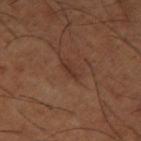Q: Is there a histopathology result?
A: catalogued during a skin exam; not biopsied
Q: What is the lesion's diameter?
A: ≈2.5 mm
Q: How was this image acquired?
A: ~15 mm crop, total-body skin-cancer survey
Q: What lighting was used for the tile?
A: cross-polarized
Q: Patient demographics?
A: male, about 65 years old
Q: Lesion location?
A: the left thigh